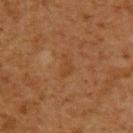<case>
<biopsy_status>not biopsied; imaged during a skin examination</biopsy_status>
<lighting>cross-polarized</lighting>
<lesion_size>
  <long_diameter_mm_approx>3.0</long_diameter_mm_approx>
</lesion_size>
<image>
  <source>total-body photography crop</source>
  <field_of_view_mm>15</field_of_view_mm>
</image>
<site>upper back</site>
<patient>
  <sex>male</sex>
  <age_approx>60</age_approx>
</patient>
</case>